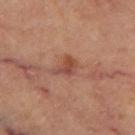• lesion size — about 3 mm
• site — the left thigh
• patient — male, in their 60s
• image source — total-body-photography crop, ~15 mm field of view